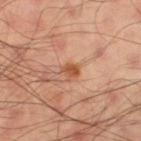Recorded during total-body skin imaging; not selected for excision or biopsy.
Captured under cross-polarized illumination.
About 2 mm across.
An algorithmic analysis of the crop reported an average lesion color of about L≈52 a*≈26 b*≈36 (CIELAB) and roughly 11 lightness units darker than nearby skin.
A male subject, about 55 years old.
On the left thigh.
A close-up tile cropped from a whole-body skin photograph, about 15 mm across.